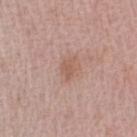Recorded during total-body skin imaging; not selected for excision or biopsy.
A roughly 15 mm field-of-view crop from a total-body skin photograph.
An algorithmic analysis of the crop reported a lesion color around L≈57 a*≈20 b*≈27 in CIELAB, about 7 CIELAB-L* units darker than the surrounding skin, and a normalized border contrast of about 5.5. The software also gave a nevus-likeness score of about 5/100.
Measured at roughly 2.5 mm in maximum diameter.
A male subject, aged around 60.
The tile uses white-light illumination.
On the right upper arm.